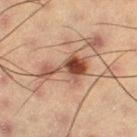Notes:
– tile lighting: cross-polarized illumination
– body site: the right thigh
– imaging modality: ~15 mm tile from a whole-body skin photo
– subject: male, about 55 years old